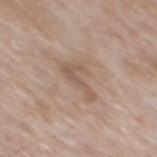biopsy_status: not biopsied; imaged during a skin examination
site: mid back
patient:
  sex: male
  age_approx: 65
image:
  source: total-body photography crop
  field_of_view_mm: 15